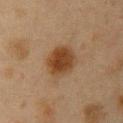Clinical impression: Recorded during total-body skin imaging; not selected for excision or biopsy. Acquisition and patient details: Imaged with cross-polarized lighting. The subject is a female aged 38 to 42. The lesion's longest dimension is about 4 mm. This image is a 15 mm lesion crop taken from a total-body photograph. The lesion is located on the right upper arm.